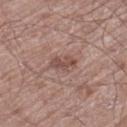Clinical impression: No biopsy was performed on this lesion — it was imaged during a full skin examination and was not determined to be concerning. Image and clinical context: Automated tile analysis of the lesion measured a classifier nevus-likeness of about 0/100 and lesion-presence confidence of about 100/100. The lesion is on the leg. The recorded lesion diameter is about 3.5 mm. A 15 mm close-up tile from a total-body photography series done for melanoma screening. The subject is a male roughly 70 years of age.